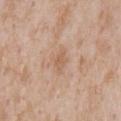{"biopsy_status": "not biopsied; imaged during a skin examination", "site": "chest", "automated_metrics": {"area_mm2_approx": 3.5, "shape_asymmetry": 0.45, "border_irregularity_0_10": 4.0, "color_variation_0_10": 1.0}, "patient": {"sex": "male", "age_approx": 65}, "image": {"source": "total-body photography crop", "field_of_view_mm": 15}}